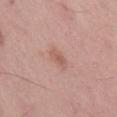Q: Is there a histopathology result?
A: no biopsy performed (imaged during a skin exam)
Q: Lesion location?
A: the mid back
Q: Patient demographics?
A: male, approximately 55 years of age
Q: What is the imaging modality?
A: ~15 mm tile from a whole-body skin photo